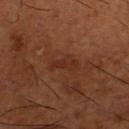* workup: catalogued during a skin exam; not biopsied
* patient: male, aged approximately 65
* location: the right lower leg
* acquisition: 15 mm crop, total-body photography